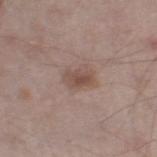{
  "image": {
    "source": "total-body photography crop",
    "field_of_view_mm": 15
  },
  "site": "right lower leg",
  "lighting": "white-light",
  "lesion_size": {
    "long_diameter_mm_approx": 3.0
  },
  "patient": {
    "sex": "male",
    "age_approx": 55
  }
}